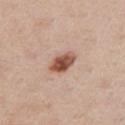Part of a total-body skin-imaging series; this lesion was reviewed on a skin check and was not flagged for biopsy. From the front of the torso. Approximately 3.5 mm at its widest. A close-up tile cropped from a whole-body skin photograph, about 15 mm across. A male patient in their mid- to late 50s.